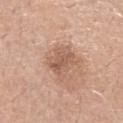Impression:
This lesion was catalogued during total-body skin photography and was not selected for biopsy.
Context:
Imaged with white-light lighting. The total-body-photography lesion software estimated a lesion–skin lightness drop of about 9 and a normalized border contrast of about 6. And it measured a border-irregularity rating of about 3.5/10, internal color variation of about 4 on a 0–10 scale, and peripheral color asymmetry of about 1.5. This image is a 15 mm lesion crop taken from a total-body photograph. The subject is a male roughly 70 years of age. The lesion's longest dimension is about 4.5 mm. On the head or neck.